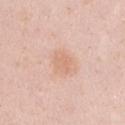Q: Is there a histopathology result?
A: imaged on a skin check; not biopsied
Q: How was this image acquired?
A: ~15 mm crop, total-body skin-cancer survey
Q: Who is the patient?
A: female, aged approximately 30
Q: How large is the lesion?
A: ≈3 mm
Q: Automated lesion metrics?
A: an average lesion color of about L≈69 a*≈21 b*≈31 (CIELAB) and a normalized border contrast of about 5; a border-irregularity rating of about 2/10, a color-variation rating of about 1.5/10, and radial color variation of about 0.5; a classifier nevus-likeness of about 15/100
Q: Where on the body is the lesion?
A: the chest